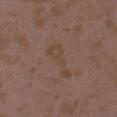Case summary:
– subject — female, approximately 35 years of age
– location — the left upper arm
– image source — total-body-photography crop, ~15 mm field of view
– lighting — white-light illumination
– lesion diameter — ~4.5 mm (longest diameter)